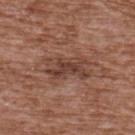Clinical impression: Recorded during total-body skin imaging; not selected for excision or biopsy. Acquisition and patient details: The lesion is on the upper back. Measured at roughly 5 mm in maximum diameter. A male subject aged approximately 75. Cropped from a whole-body photographic skin survey; the tile spans about 15 mm. The tile uses white-light illumination. The total-body-photography lesion software estimated a classifier nevus-likeness of about 0/100.